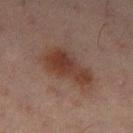<lesion>
  <biopsy_status>not biopsied; imaged during a skin examination</biopsy_status>
  <automated_metrics>
    <area_mm2_approx>15.0</area_mm2_approx>
    <eccentricity>0.9</eccentricity>
    <shape_asymmetry>0.3</shape_asymmetry>
    <vs_skin_darker_L>8.0</vs_skin_darker_L>
    <vs_skin_contrast_norm>8.5</vs_skin_contrast_norm>
  </automated_metrics>
  <lesion_size>
    <long_diameter_mm_approx>6.5</long_diameter_mm_approx>
  </lesion_size>
  <site>left thigh</site>
  <lighting>cross-polarized</lighting>
  <patient>
    <sex>male</sex>
    <age_approx>45</age_approx>
  </patient>
  <image>
    <source>total-body photography crop</source>
    <field_of_view_mm>15</field_of_view_mm>
  </image>
</lesion>